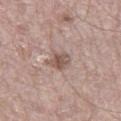biopsy status: no biopsy performed (imaged during a skin exam) | lesion size: about 2.5 mm | subject: male, aged approximately 70 | TBP lesion metrics: a footprint of about 4.5 mm², an outline eccentricity of about 0.5 (0 = round, 1 = elongated), and a symmetry-axis asymmetry near 0.2; a mean CIELAB color near L≈53 a*≈17 b*≈23, about 11 CIELAB-L* units darker than the surrounding skin, and a normalized lesion–skin contrast near 7.5; a border-irregularity index near 2/10, a within-lesion color-variation index near 3.5/10, and peripheral color asymmetry of about 1; a classifier nevus-likeness of about 0/100 and lesion-presence confidence of about 100/100 | illumination: white-light illumination | image: ~15 mm tile from a whole-body skin photo | site: the right thigh.